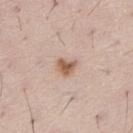<record>
  <biopsy_status>not biopsied; imaged during a skin examination</biopsy_status>
  <automated_metrics>
    <cielab_L>59</cielab_L>
    <cielab_a>19</cielab_a>
    <cielab_b>30</cielab_b>
    <vs_skin_contrast_norm>8.5</vs_skin_contrast_norm>
    <border_irregularity_0_10>3.0</border_irregularity_0_10>
    <peripheral_color_asymmetry>1.0</peripheral_color_asymmetry>
  </automated_metrics>
  <patient>
    <sex>male</sex>
    <age_approx>50</age_approx>
  </patient>
  <site>left thigh</site>
  <lesion_size>
    <long_diameter_mm_approx>2.5</long_diameter_mm_approx>
  </lesion_size>
  <image>
    <source>total-body photography crop</source>
    <field_of_view_mm>15</field_of_view_mm>
  </image>
</record>